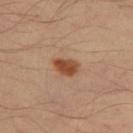  biopsy_status: not biopsied; imaged during a skin examination
  site: left thigh
  patient:
    sex: male
    age_approx: 40
  image:
    source: total-body photography crop
    field_of_view_mm: 15